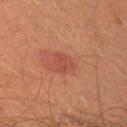{
  "biopsy_status": "not biopsied; imaged during a skin examination",
  "patient": {
    "sex": "male",
    "age_approx": 45
  },
  "site": "arm",
  "image": {
    "source": "total-body photography crop",
    "field_of_view_mm": 15
  }
}